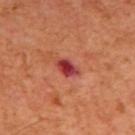  biopsy_status: not biopsied; imaged during a skin examination
  automated_metrics:
    area_mm2_approx: 4.0
    eccentricity: 0.8
    shape_asymmetry: 0.25
    cielab_L: 40
    cielab_a: 37
    cielab_b: 27
    vs_skin_contrast_norm: 11.5
    border_irregularity_0_10: 2.0
    peripheral_color_asymmetry: 2.5
  lighting: cross-polarized
  patient:
    sex: male
    age_approx: 65
  image:
    source: total-body photography crop
    field_of_view_mm: 15
  lesion_size:
    long_diameter_mm_approx: 3.0
  site: mid back